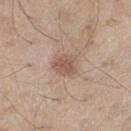{
  "biopsy_status": "not biopsied; imaged during a skin examination",
  "patient": {
    "sex": "male",
    "age_approx": 60
  },
  "automated_metrics": {
    "area_mm2_approx": 5.5,
    "shape_asymmetry": 0.2,
    "lesion_detection_confidence_0_100": 100
  },
  "lighting": "white-light",
  "image": {
    "source": "total-body photography crop",
    "field_of_view_mm": 15
  },
  "site": "right thigh",
  "lesion_size": {
    "long_diameter_mm_approx": 3.0
  }
}